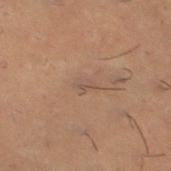The lesion was tiled from a total-body skin photograph and was not biopsied.
Measured at roughly 2.5 mm in maximum diameter.
Captured under cross-polarized illumination.
A male subject, aged 28 to 32.
A roughly 15 mm field-of-view crop from a total-body skin photograph.
On the left lower leg.